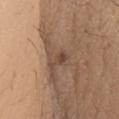Findings:
• notes: total-body-photography surveillance lesion; no biopsy
• site: the chest
• diameter: ~3 mm (longest diameter)
• image-analysis metrics: a footprint of about 3 mm², an outline eccentricity of about 0.9 (0 = round, 1 = elongated), and a symmetry-axis asymmetry near 0.45; an average lesion color of about L≈45 a*≈17 b*≈27 (CIELAB), a lesion–skin lightness drop of about 9, and a normalized lesion–skin contrast near 7.5; a classifier nevus-likeness of about 0/100 and a detector confidence of about 95 out of 100 that the crop contains a lesion
• patient: female, aged approximately 40
• illumination: white-light
• acquisition: 15 mm crop, total-body photography